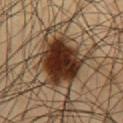| key | value |
|---|---|
| size | about 6.5 mm |
| patient | male, about 60 years old |
| anatomic site | the abdomen |
| lighting | cross-polarized |
| image | ~15 mm crop, total-body skin-cancer survey |